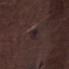Impression: The lesion was tiled from a total-body skin photograph and was not biopsied. Clinical summary: Located on the right thigh. A lesion tile, about 15 mm wide, cut from a 3D total-body photograph. A male subject in their mid-70s.